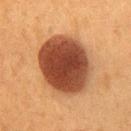{
  "biopsy_status": "not biopsied; imaged during a skin examination",
  "lesion_size": {
    "long_diameter_mm_approx": 7.5
  },
  "patient": {
    "sex": "female",
    "age_approx": 50
  },
  "lighting": "cross-polarized",
  "site": "front of the torso",
  "image": {
    "source": "total-body photography crop",
    "field_of_view_mm": 15
  }
}